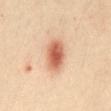Acquisition and patient details:
Cropped from a whole-body photographic skin survey; the tile spans about 15 mm. Automated tile analysis of the lesion measured a footprint of about 10 mm² and a shape eccentricity near 0.7. The analysis additionally found an automated nevus-likeness rating near 100 out of 100. Longest diameter approximately 4 mm. From the abdomen. The subject is a female about 40 years old. Imaged with cross-polarized lighting.